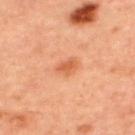A female patient roughly 45 years of age. This image is a 15 mm lesion crop taken from a total-body photograph. The lesion is located on the upper back. Automated image analysis of the tile measured a footprint of about 4 mm² and a shape-asymmetry score of about 0.25 (0 = symmetric). The analysis additionally found an average lesion color of about L≈60 a*≈29 b*≈40 (CIELAB), roughly 10 lightness units darker than nearby skin, and a normalized lesion–skin contrast near 6.5. And it measured a nevus-likeness score of about 60/100 and a detector confidence of about 100 out of 100 that the crop contains a lesion. Approximately 2.5 mm at its widest.